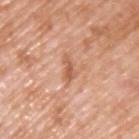Case summary:
• biopsy status · catalogued during a skin exam; not biopsied
• image source · 15 mm crop, total-body photography
• lighting · white-light illumination
• patient · male, about 60 years old
• anatomic site · the upper back
• TBP lesion metrics · a symmetry-axis asymmetry near 0.4; an average lesion color of about L≈59 a*≈24 b*≈33 (CIELAB), a lesion–skin lightness drop of about 10, and a normalized lesion–skin contrast near 6.5; a detector confidence of about 100 out of 100 that the crop contains a lesion